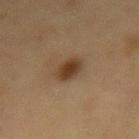imaging modality = total-body-photography crop, ~15 mm field of view
anatomic site = the mid back
image-analysis metrics = an average lesion color of about L≈33 a*≈15 b*≈27 (CIELAB), roughly 10 lightness units darker than nearby skin, and a normalized border contrast of about 10; a border-irregularity index near 1.5/10, a color-variation rating of about 3/10, and a peripheral color-asymmetry measure near 1
subject = female, approximately 55 years of age
tile lighting = cross-polarized illumination
size = ~3 mm (longest diameter)